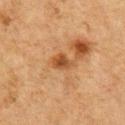  biopsy_status: not biopsied; imaged during a skin examination
  image:
    source: total-body photography crop
    field_of_view_mm: 15
  site: chest
  patient:
    sex: male
    age_approx: 75